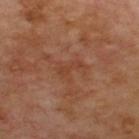Q: Was a biopsy performed?
A: catalogued during a skin exam; not biopsied
Q: What is the lesion's diameter?
A: ≈3.5 mm
Q: Where on the body is the lesion?
A: the back
Q: What is the imaging modality?
A: 15 mm crop, total-body photography
Q: Patient demographics?
A: male, approximately 70 years of age
Q: What did automated image analysis measure?
A: an area of roughly 5 mm², a shape eccentricity near 0.85, and a symmetry-axis asymmetry near 0.6; a classifier nevus-likeness of about 0/100 and a lesion-detection confidence of about 100/100
Q: What lighting was used for the tile?
A: cross-polarized illumination